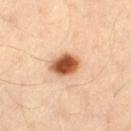Q: Was this lesion biopsied?
A: catalogued during a skin exam; not biopsied
Q: How was this image acquired?
A: 15 mm crop, total-body photography
Q: Patient demographics?
A: male, roughly 40 years of age
Q: Lesion location?
A: the left thigh